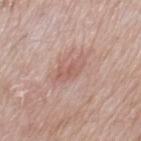Q: What are the patient's age and sex?
A: male, aged approximately 70
Q: What is the lesion's diameter?
A: ≈4 mm
Q: What is the imaging modality?
A: 15 mm crop, total-body photography
Q: What lighting was used for the tile?
A: white-light illumination
Q: Where on the body is the lesion?
A: the chest